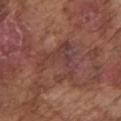biopsy status: no biopsy performed (imaged during a skin exam)
body site: the left upper arm
automated metrics: a footprint of about 12 mm², an outline eccentricity of about 0.3 (0 = round, 1 = elongated), and a symmetry-axis asymmetry near 0.75; an automated nevus-likeness rating near 0 out of 100
subject: male, in their mid-70s
illumination: white-light
size: about 5 mm
image: total-body-photography crop, ~15 mm field of view